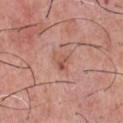| key | value |
|---|---|
| notes | imaged on a skin check; not biopsied |
| diameter | about 2.5 mm |
| site | the chest |
| TBP lesion metrics | an average lesion color of about L≈55 a*≈25 b*≈29 (CIELAB), roughly 8 lightness units darker than nearby skin, and a normalized lesion–skin contrast near 6; a border-irregularity rating of about 3/10 and a color-variation rating of about 4.5/10 |
| imaging modality | 15 mm crop, total-body photography |
| patient | male, in their mid-50s |
| lighting | white-light |